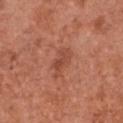Imaged during a routine full-body skin examination; the lesion was not biopsied and no histopathology is available. The subject is a female about 50 years old. A lesion tile, about 15 mm wide, cut from a 3D total-body photograph. The lesion is on the chest. Captured under white-light illumination. Automated tile analysis of the lesion measured a lesion color around L≈48 a*≈27 b*≈32 in CIELAB, roughly 8 lightness units darker than nearby skin, and a normalized lesion–skin contrast near 6. The analysis additionally found an automated nevus-likeness rating near 5 out of 100 and a lesion-detection confidence of about 100/100. The lesion's longest dimension is about 4 mm.